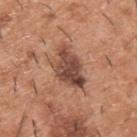Recorded during total-body skin imaging; not selected for excision or biopsy.
Approximately 5 mm at its widest.
Imaged with white-light lighting.
A male patient, aged 38–42.
The lesion is on the upper back.
A roughly 15 mm field-of-view crop from a total-body skin photograph.